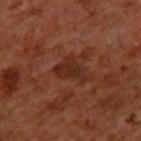workup = imaged on a skin check; not biopsied | anatomic site = the back | lighting = cross-polarized | subject = male, aged approximately 50 | acquisition = total-body-photography crop, ~15 mm field of view.